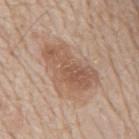<lesion>
  <biopsy_status>not biopsied; imaged during a skin examination</biopsy_status>
  <patient>
    <sex>male</sex>
    <age_approx>80</age_approx>
  </patient>
  <site>mid back</site>
  <automated_metrics>
    <border_irregularity_0_10>3.5</border_irregularity_0_10>
    <color_variation_0_10>4.5</color_variation_0_10>
    <nevus_likeness_0_100>15</nevus_likeness_0_100>
    <lesion_detection_confidence_0_100>100</lesion_detection_confidence_0_100>
  </automated_metrics>
  <image>
    <source>total-body photography crop</source>
    <field_of_view_mm>15</field_of_view_mm>
  </image>
</lesion>